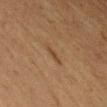Findings:
• notes · catalogued during a skin exam; not biopsied
• subject · female, aged approximately 50
• lesion size · ~3 mm (longest diameter)
• site · the arm
• image · 15 mm crop, total-body photography
• image-analysis metrics · a lesion area of about 3 mm², an eccentricity of roughly 0.9, and a shape-asymmetry score of about 0.25 (0 = symmetric); border irregularity of about 3 on a 0–10 scale, a color-variation rating of about 1/10, and a peripheral color-asymmetry measure near 0; an automated nevus-likeness rating near 10 out of 100 and a detector confidence of about 100 out of 100 that the crop contains a lesion